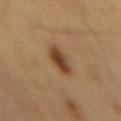{
  "biopsy_status": "not biopsied; imaged during a skin examination",
  "automated_metrics": {
    "border_irregularity_0_10": 1.5,
    "lesion_detection_confidence_0_100": 100
  },
  "patient": {
    "sex": "male",
    "age_approx": 60
  },
  "image": {
    "source": "total-body photography crop",
    "field_of_view_mm": 15
  },
  "site": "mid back",
  "lighting": "cross-polarized"
}